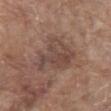follow-up: total-body-photography surveillance lesion; no biopsy
site: the front of the torso
diameter: about 6 mm
imaging modality: 15 mm crop, total-body photography
automated metrics: an area of roughly 17 mm², a shape eccentricity near 0.7, and two-axis asymmetry of about 0.4; a nevus-likeness score of about 0/100 and a detector confidence of about 100 out of 100 that the crop contains a lesion
lighting: white-light
subject: male, roughly 80 years of age